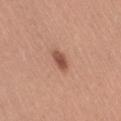Case summary:
• follow-up — total-body-photography surveillance lesion; no biopsy